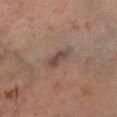Assessment:
The lesion was photographed on a routine skin check and not biopsied; there is no pathology result.